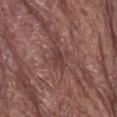The tile uses white-light illumination. The lesion-visualizer software estimated a mean CIELAB color near L≈39 a*≈21 b*≈21 and about 7 CIELAB-L* units darker than the surrounding skin. And it measured internal color variation of about 1 on a 0–10 scale and peripheral color asymmetry of about 0.5. A male subject, aged approximately 80. Longest diameter approximately 3 mm. Located on the head or neck. A 15 mm crop from a total-body photograph taken for skin-cancer surveillance.